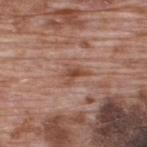Recorded during total-body skin imaging; not selected for excision or biopsy.
A 15 mm crop from a total-body photograph taken for skin-cancer surveillance.
The lesion's longest dimension is about 3 mm.
A male subject in their 70s.
Automated image analysis of the tile measured a footprint of about 4 mm², an outline eccentricity of about 0.8 (0 = round, 1 = elongated), and two-axis asymmetry of about 0.4. The software also gave a classifier nevus-likeness of about 0/100 and a lesion-detection confidence of about 100/100.
From the upper back.